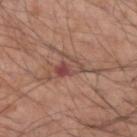A male patient about 55 years old.
Cropped from a whole-body photographic skin survey; the tile spans about 15 mm.
Longest diameter approximately 6 mm.
The total-body-photography lesion software estimated a lesion area of about 9.5 mm², an eccentricity of roughly 0.8, and a shape-asymmetry score of about 0.55 (0 = symmetric). It also reported a mean CIELAB color near L≈48 a*≈21 b*≈25, a lesion–skin lightness drop of about 9, and a lesion-to-skin contrast of about 6.5 (normalized; higher = more distinct). And it measured a color-variation rating of about 8.5/10. And it measured a classifier nevus-likeness of about 0/100 and a lesion-detection confidence of about 70/100.
Captured under white-light illumination.
From the right forearm.